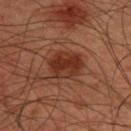{
  "biopsy_status": "not biopsied; imaged during a skin examination",
  "lighting": "cross-polarized",
  "patient": {
    "sex": "male",
    "age_approx": 45
  },
  "site": "upper back",
  "automated_metrics": {
    "area_mm2_approx": 11.0,
    "eccentricity": 0.6,
    "cielab_L": 31,
    "cielab_a": 24,
    "cielab_b": 28,
    "vs_skin_darker_L": 9.0,
    "vs_skin_contrast_norm": 9.0,
    "nevus_likeness_0_100": 90,
    "lesion_detection_confidence_0_100": 100
  },
  "image": {
    "source": "total-body photography crop",
    "field_of_view_mm": 15
  },
  "lesion_size": {
    "long_diameter_mm_approx": 4.0
  }
}